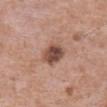Impression: Captured during whole-body skin photography for melanoma surveillance; the lesion was not biopsied. Context: The tile uses white-light illumination. The lesion is on the front of the torso. This image is a 15 mm lesion crop taken from a total-body photograph. About 3.5 mm across. A male patient, aged approximately 70.